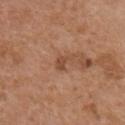Part of a total-body skin-imaging series; this lesion was reviewed on a skin check and was not flagged for biopsy. About 2.5 mm across. A male patient, in their mid- to late 70s. This is a white-light tile. Automated image analysis of the tile measured a footprint of about 3 mm², a shape eccentricity near 0.8, and a shape-asymmetry score of about 0.45 (0 = symmetric). A roughly 15 mm field-of-view crop from a total-body skin photograph. From the chest.